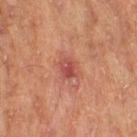Case summary:
- notes · total-body-photography surveillance lesion; no biopsy
- image source · 15 mm crop, total-body photography
- size · ~3 mm (longest diameter)
- lighting · cross-polarized illumination
- patient · male, roughly 70 years of age
- image-analysis metrics · a border-irregularity rating of about 2/10, a within-lesion color-variation index near 4/10, and radial color variation of about 1.5; an automated nevus-likeness rating near 5 out of 100 and a detector confidence of about 100 out of 100 that the crop contains a lesion
- anatomic site · the right thigh